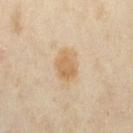No biopsy was performed on this lesion — it was imaged during a full skin examination and was not determined to be concerning. The tile uses cross-polarized illumination. The lesion's longest dimension is about 3.5 mm. Located on the lower back. A female subject, aged 33 to 37. The lesion-visualizer software estimated a footprint of about 7.5 mm², a shape eccentricity near 0.7, and a symmetry-axis asymmetry near 0.2. The analysis additionally found a classifier nevus-likeness of about 70/100 and a detector confidence of about 100 out of 100 that the crop contains a lesion. Cropped from a total-body skin-imaging series; the visible field is about 15 mm.